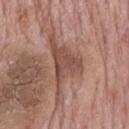notes — total-body-photography surveillance lesion; no biopsy
illumination — white-light illumination
subject — male, about 75 years old
location — the mid back
image-analysis metrics — a footprint of about 14 mm², a shape eccentricity near 0.8, and a shape-asymmetry score of about 0.65 (0 = symmetric); an average lesion color of about L≈49 a*≈21 b*≈26 (CIELAB) and a lesion–skin lightness drop of about 9; a peripheral color-asymmetry measure near 1; an automated nevus-likeness rating near 0 out of 100 and a detector confidence of about 65 out of 100 that the crop contains a lesion
imaging modality — ~15 mm tile from a whole-body skin photo
diameter — about 8 mm